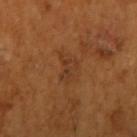Findings:
• notes — total-body-photography surveillance lesion; no biopsy
• diameter — ~3.5 mm (longest diameter)
• imaging modality — 15 mm crop, total-body photography
• automated metrics — a lesion area of about 6.5 mm², a shape eccentricity near 0.65, and a shape-asymmetry score of about 0.4 (0 = symmetric); a classifier nevus-likeness of about 0/100 and a detector confidence of about 100 out of 100 that the crop contains a lesion
• site — the right upper arm
• patient — male, in their mid- to late 60s